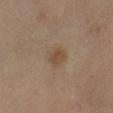Captured during whole-body skin photography for melanoma surveillance; the lesion was not biopsied. The lesion is on the right lower leg. Captured under cross-polarized illumination. A roughly 15 mm field-of-view crop from a total-body skin photograph. Automated tile analysis of the lesion measured a lesion color around L≈40 a*≈13 b*≈26 in CIELAB, a lesion–skin lightness drop of about 6, and a normalized border contrast of about 6.5. It also reported a border-irregularity index near 1.5/10, internal color variation of about 1.5 on a 0–10 scale, and radial color variation of about 0.5. It also reported an automated nevus-likeness rating near 60 out of 100 and a lesion-detection confidence of about 100/100. A female subject aged 53 to 57.